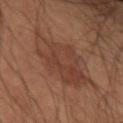No biopsy was performed on this lesion — it was imaged during a full skin examination and was not determined to be concerning. A male patient, about 55 years old. About 7.5 mm across. Captured under cross-polarized illumination. The lesion is on the left forearm. A region of skin cropped from a whole-body photographic capture, roughly 15 mm wide.